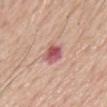Imaged during a routine full-body skin examination; the lesion was not biopsied and no histopathology is available.
Imaged with white-light lighting.
The subject is a male roughly 65 years of age.
The total-body-photography lesion software estimated a mean CIELAB color near L≈55 a*≈29 b*≈22 and roughly 14 lightness units darker than nearby skin. And it measured a nevus-likeness score of about 0/100.
The lesion is on the back.
A region of skin cropped from a whole-body photographic capture, roughly 15 mm wide.